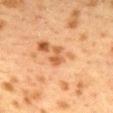This lesion was catalogued during total-body skin photography and was not selected for biopsy. Cropped from a total-body skin-imaging series; the visible field is about 15 mm. The recorded lesion diameter is about 2.5 mm. A female patient, aged around 40. From the mid back.